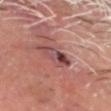follow-up: imaged on a skin check; not biopsied | site: the head or neck | image: ~15 mm tile from a whole-body skin photo | lesion size: about 3.5 mm | tile lighting: cross-polarized | automated metrics: an area of roughly 7 mm² and a shape-asymmetry score of about 0.35 (0 = symmetric); a border-irregularity rating of about 4/10, internal color variation of about 10 on a 0–10 scale, and a peripheral color-asymmetry measure near 5 | subject: male, approximately 60 years of age.